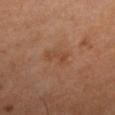follow-up = imaged on a skin check; not biopsied
patient = female, approximately 50 years of age
acquisition = ~15 mm tile from a whole-body skin photo
image-analysis metrics = an area of roughly 5 mm² and two-axis asymmetry of about 0.35
lesion diameter = ~3.5 mm (longest diameter)
tile lighting = cross-polarized illumination